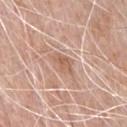Case summary:
• notes — total-body-photography surveillance lesion; no biopsy
• subject — male, aged 78 to 82
• image — ~15 mm tile from a whole-body skin photo
• illumination — white-light illumination
• body site — the chest